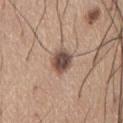The lesion was photographed on a routine skin check and not biopsied; there is no pathology result.
Measured at roughly 3.5 mm in maximum diameter.
From the front of the torso.
A male patient, approximately 65 years of age.
Cropped from a total-body skin-imaging series; the visible field is about 15 mm.